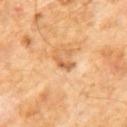Assessment:
Captured during whole-body skin photography for melanoma surveillance; the lesion was not biopsied.
Acquisition and patient details:
On the abdomen. The lesion's longest dimension is about 2.5 mm. A 15 mm close-up tile from a total-body photography series done for melanoma screening. The tile uses cross-polarized illumination. A male patient aged approximately 60. An algorithmic analysis of the crop reported an outline eccentricity of about 0.9 (0 = round, 1 = elongated). It also reported a lesion color around L≈59 a*≈23 b*≈40 in CIELAB and a lesion-to-skin contrast of about 7 (normalized; higher = more distinct). It also reported border irregularity of about 5 on a 0–10 scale, a within-lesion color-variation index near 0/10, and radial color variation of about 0. The analysis additionally found a nevus-likeness score of about 0/100.